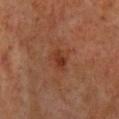| field | value |
|---|---|
| follow-up | total-body-photography surveillance lesion; no biopsy |
| site | the mid back |
| subject | male, aged 58–62 |
| diameter | ~3 mm (longest diameter) |
| image | ~15 mm crop, total-body skin-cancer survey |
| tile lighting | cross-polarized |